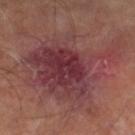Captured during whole-body skin photography for melanoma surveillance; the lesion was not biopsied. Imaged with cross-polarized lighting. The lesion's longest dimension is about 12 mm. The lesion is on the right lower leg. The total-body-photography lesion software estimated a border-irregularity rating of about 8/10 and peripheral color asymmetry of about 1.5. The analysis additionally found a classifier nevus-likeness of about 0/100 and lesion-presence confidence of about 100/100. A 15 mm close-up tile from a total-body photography series done for melanoma screening. The patient is a male aged 63 to 67.